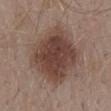<tbp_lesion>
  <biopsy_status>not biopsied; imaged during a skin examination</biopsy_status>
  <site>mid back</site>
  <lesion_size>
    <long_diameter_mm_approx>9.0</long_diameter_mm_approx>
  </lesion_size>
  <patient>
    <sex>male</sex>
    <age_approx>55</age_approx>
  </patient>
  <image>
    <source>total-body photography crop</source>
    <field_of_view_mm>15</field_of_view_mm>
  </image>
  <lighting>white-light</lighting>
  <automated_metrics>
    <border_irregularity_0_10>2.5</border_irregularity_0_10>
    <color_variation_0_10>4.5</color_variation_0_10>
    <peripheral_color_asymmetry>1.5</peripheral_color_asymmetry>
    <nevus_likeness_0_100>90</nevus_likeness_0_100>
    <lesion_detection_confidence_0_100>100</lesion_detection_confidence_0_100>
  </automated_metrics>
</tbp_lesion>